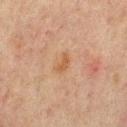This image is a 15 mm lesion crop taken from a total-body photograph.
Captured under cross-polarized illumination.
A male patient, in their mid- to late 60s.
The recorded lesion diameter is about 2.5 mm.
Located on the mid back.